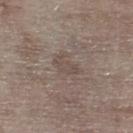* workup: imaged on a skin check; not biopsied
* subject: male, aged 68–72
* image source: ~15 mm crop, total-body skin-cancer survey
* location: the right lower leg